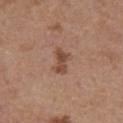Image and clinical context: Captured under white-light illumination. An algorithmic analysis of the crop reported border irregularity of about 4.5 on a 0–10 scale and peripheral color asymmetry of about 1. And it measured an automated nevus-likeness rating near 75 out of 100 and a detector confidence of about 100 out of 100 that the crop contains a lesion. Longest diameter approximately 3 mm. The lesion is located on the front of the torso. The patient is a female in their mid- to late 60s. A 15 mm close-up extracted from a 3D total-body photography capture.